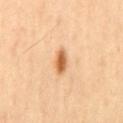| feature | finding |
|---|---|
| workup | imaged on a skin check; not biopsied |
| patient | male, aged 53 to 57 |
| anatomic site | the mid back |
| image source | total-body-photography crop, ~15 mm field of view |
| tile lighting | cross-polarized |